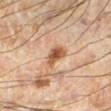The lesion was tiled from a total-body skin photograph and was not biopsied. Automated image analysis of the tile measured a footprint of about 5.5 mm², an outline eccentricity of about 0.75 (0 = round, 1 = elongated), and two-axis asymmetry of about 0.35. And it measured a lesion color around L≈41 a*≈17 b*≈27 in CIELAB and a normalized lesion–skin contrast near 8.5. It also reported a border-irregularity rating of about 3.5/10, a color-variation rating of about 3/10, and a peripheral color-asymmetry measure near 0.5. A roughly 15 mm field-of-view crop from a total-body skin photograph. A male subject, aged 58 to 62. The recorded lesion diameter is about 3 mm. On the left leg. This is a cross-polarized tile.